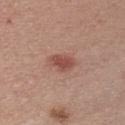Notes:
- follow-up · total-body-photography surveillance lesion; no biopsy
- lesion diameter · about 3.5 mm
- image source · total-body-photography crop, ~15 mm field of view
- patient · male, roughly 30 years of age
- illumination · white-light